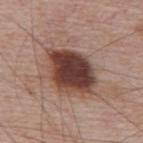Q: Is there a histopathology result?
A: imaged on a skin check; not biopsied
Q: How was this image acquired?
A: 15 mm crop, total-body photography
Q: What are the patient's age and sex?
A: male, about 65 years old
Q: What is the anatomic site?
A: the upper back
Q: How large is the lesion?
A: ≈6.5 mm
Q: How was the tile lit?
A: white-light illumination